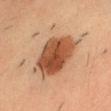Q: Is there a histopathology result?
A: total-body-photography surveillance lesion; no biopsy
Q: What is the anatomic site?
A: the head or neck
Q: What did automated image analysis measure?
A: an area of roughly 22 mm² and two-axis asymmetry of about 0.15; a lesion color around L≈45 a*≈22 b*≈32 in CIELAB, a lesion–skin lightness drop of about 15, and a normalized border contrast of about 10.5; border irregularity of about 2 on a 0–10 scale, a color-variation rating of about 5.5/10, and radial color variation of about 2
Q: How large is the lesion?
A: ~7 mm (longest diameter)
Q: Illumination type?
A: cross-polarized illumination
Q: What kind of image is this?
A: total-body-photography crop, ~15 mm field of view
Q: Who is the patient?
A: male, approximately 35 years of age